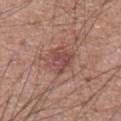No biopsy was performed on this lesion — it was imaged during a full skin examination and was not determined to be concerning. The subject is a male about 75 years old. Imaged with white-light lighting. A close-up tile cropped from a whole-body skin photograph, about 15 mm across. The lesion-visualizer software estimated an average lesion color of about L≈48 a*≈23 b*≈23 (CIELAB), a lesion–skin lightness drop of about 8, and a lesion-to-skin contrast of about 6 (normalized; higher = more distinct). It also reported a classifier nevus-likeness of about 0/100 and a lesion-detection confidence of about 100/100. Located on the abdomen. Measured at roughly 3.5 mm in maximum diameter.